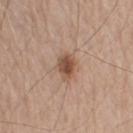Imaged during a routine full-body skin examination; the lesion was not biopsied and no histopathology is available.
A region of skin cropped from a whole-body photographic capture, roughly 15 mm wide.
An algorithmic analysis of the crop reported an area of roughly 6 mm², an outline eccentricity of about 0.7 (0 = round, 1 = elongated), and a shape-asymmetry score of about 0.2 (0 = symmetric). The software also gave a lesion color around L≈51 a*≈19 b*≈29 in CIELAB, roughly 12 lightness units darker than nearby skin, and a lesion-to-skin contrast of about 9 (normalized; higher = more distinct). And it measured a border-irregularity index near 2/10, internal color variation of about 4 on a 0–10 scale, and radial color variation of about 1.
The tile uses white-light illumination.
Located on the right upper arm.
The lesion's longest dimension is about 3.5 mm.
A male subject, about 75 years old.